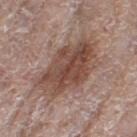The lesion was tiled from a total-body skin photograph and was not biopsied. A female patient, roughly 75 years of age. The lesion is located on the left thigh. Cropped from a whole-body photographic skin survey; the tile spans about 15 mm. Longest diameter approximately 7.5 mm. The tile uses white-light illumination.